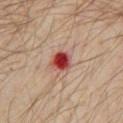  biopsy_status: not biopsied; imaged during a skin examination
  lighting: cross-polarized
  image:
    source: total-body photography crop
    field_of_view_mm: 15
  site: front of the torso
  lesion_size:
    long_diameter_mm_approx: 2.5
  patient:
    sex: male
    age_approx: 30